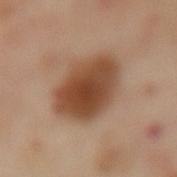The lesion was tiled from a total-body skin photograph and was not biopsied.
A 15 mm close-up tile from a total-body photography series done for melanoma screening.
Captured under cross-polarized illumination.
An algorithmic analysis of the crop reported an average lesion color of about L≈47 a*≈20 b*≈32 (CIELAB), a lesion–skin lightness drop of about 14, and a normalized border contrast of about 10.5.
A female patient in their mid-50s.
Located on the lower back.
Longest diameter approximately 6 mm.